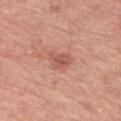Notes:
- follow-up · total-body-photography surveillance lesion; no biopsy
- diameter · about 3 mm
- subject · male, aged 73 to 77
- anatomic site · the left thigh
- imaging modality · ~15 mm crop, total-body skin-cancer survey
- automated metrics · roughly 9 lightness units darker than nearby skin and a normalized lesion–skin contrast near 6
- lighting · white-light illumination